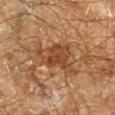follow-up: no biopsy performed (imaged during a skin exam)
subject: male, about 60 years old
automated lesion analysis: an average lesion color of about L≈34 a*≈18 b*≈27 (CIELAB), a lesion–skin lightness drop of about 8, and a lesion-to-skin contrast of about 7.5 (normalized; higher = more distinct)
image: total-body-photography crop, ~15 mm field of view
diameter: ≈6.5 mm
body site: the right lower leg